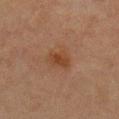Q: Was this lesion biopsied?
A: no biopsy performed (imaged during a skin exam)
Q: Illumination type?
A: cross-polarized
Q: What kind of image is this?
A: total-body-photography crop, ~15 mm field of view
Q: Lesion size?
A: about 3 mm
Q: What is the anatomic site?
A: the chest
Q: Patient demographics?
A: female, about 60 years old